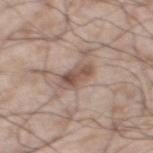Acquisition and patient details:
A 15 mm crop from a total-body photograph taken for skin-cancer surveillance. From the left thigh. A male patient, aged 58–62. The tile uses white-light illumination.